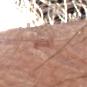Recorded during total-body skin imaging; not selected for excision or biopsy. A male patient aged around 70. On the left forearm. A 15 mm close-up tile from a total-body photography series done for melanoma screening. Measured at roughly 3 mm in maximum diameter. Imaged with white-light lighting.